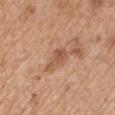Clinical impression: Recorded during total-body skin imaging; not selected for excision or biopsy. Clinical summary: Cropped from a total-body skin-imaging series; the visible field is about 15 mm. From the right upper arm. The patient is a male aged approximately 65.